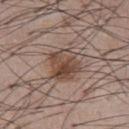Clinical impression: The lesion was tiled from a total-body skin photograph and was not biopsied. Context: Cropped from a total-body skin-imaging series; the visible field is about 15 mm. Measured at roughly 4 mm in maximum diameter. The lesion-visualizer software estimated a shape eccentricity near 0.4 and a symmetry-axis asymmetry near 0.15. The software also gave a mean CIELAB color near L≈46 a*≈17 b*≈25, about 11 CIELAB-L* units darker than the surrounding skin, and a lesion-to-skin contrast of about 8.5 (normalized; higher = more distinct). The software also gave a nevus-likeness score of about 65/100 and lesion-presence confidence of about 100/100. The subject is a male approximately 55 years of age. Located on the chest.